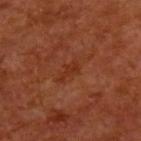- follow-up: total-body-photography surveillance lesion; no biopsy
- lesion size: about 2.5 mm
- tile lighting: cross-polarized illumination
- imaging modality: ~15 mm tile from a whole-body skin photo
- TBP lesion metrics: two-axis asymmetry of about 0.45; a border-irregularity index near 5/10, a color-variation rating of about 0/10, and a peripheral color-asymmetry measure near 0; a nevus-likeness score of about 0/100
- site: the upper back
- patient: male, aged 63 to 67